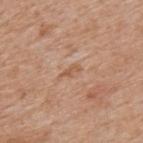Part of a total-body skin-imaging series; this lesion was reviewed on a skin check and was not flagged for biopsy. Cropped from a whole-body photographic skin survey; the tile spans about 15 mm. The lesion's longest dimension is about 2.5 mm. The patient is a male in their mid- to late 60s. Located on the upper back.